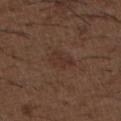Captured during whole-body skin photography for melanoma surveillance; the lesion was not biopsied. A close-up tile cropped from a whole-body skin photograph, about 15 mm across. Located on the upper back. A male subject aged 48 to 52. Measured at roughly 3.5 mm in maximum diameter. Automated tile analysis of the lesion measured an area of roughly 5.5 mm², an outline eccentricity of about 0.8 (0 = round, 1 = elongated), and a symmetry-axis asymmetry near 0.3. The software also gave an average lesion color of about L≈32 a*≈17 b*≈24 (CIELAB), a lesion–skin lightness drop of about 5, and a normalized border contrast of about 5. The software also gave border irregularity of about 3 on a 0–10 scale, a color-variation rating of about 1.5/10, and radial color variation of about 0.5. The analysis additionally found a nevus-likeness score of about 0/100 and lesion-presence confidence of about 100/100. Imaged with white-light lighting.